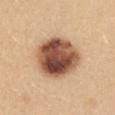Q: Is there a histopathology result?
A: catalogued during a skin exam; not biopsied
Q: Illumination type?
A: white-light illumination
Q: What is the imaging modality?
A: ~15 mm tile from a whole-body skin photo
Q: Automated lesion metrics?
A: an area of roughly 24 mm²; an automated nevus-likeness rating near 95 out of 100 and a detector confidence of about 100 out of 100 that the crop contains a lesion
Q: What are the patient's age and sex?
A: female, aged around 25
Q: Lesion location?
A: the mid back